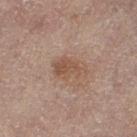| feature | finding |
|---|---|
| follow-up | no biopsy performed (imaged during a skin exam) |
| acquisition | total-body-photography crop, ~15 mm field of view |
| illumination | cross-polarized |
| image-analysis metrics | a border-irregularity rating of about 3.5/10, a within-lesion color-variation index near 3/10, and a peripheral color-asymmetry measure near 1; a classifier nevus-likeness of about 15/100 |
| anatomic site | the right leg |
| lesion size | about 4 mm |
| patient | female, aged approximately 80 |